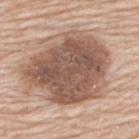An algorithmic analysis of the crop reported border irregularity of about 2.5 on a 0–10 scale, a within-lesion color-variation index near 5/10, and a peripheral color-asymmetry measure near 1.5. The software also gave an automated nevus-likeness rating near 30 out of 100 and a detector confidence of about 100 out of 100 that the crop contains a lesion.
A close-up tile cropped from a whole-body skin photograph, about 15 mm across.
The recorded lesion diameter is about 9.5 mm.
A male patient approximately 80 years of age.
Imaged with white-light lighting.
The lesion is located on the upper back.